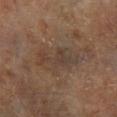Assessment: Recorded during total-body skin imaging; not selected for excision or biopsy. Image and clinical context: A male subject, in their mid-80s or older. The lesion is located on the right lower leg. A 15 mm close-up tile from a total-body photography series done for melanoma screening. Automated tile analysis of the lesion measured two-axis asymmetry of about 0.45. And it measured an automated nevus-likeness rating near 0 out of 100. The lesion's longest dimension is about 5 mm. The tile uses cross-polarized illumination.